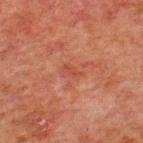Q: Is there a histopathology result?
A: imaged on a skin check; not biopsied
Q: Automated lesion metrics?
A: an outline eccentricity of about 0.85 (0 = round, 1 = elongated); internal color variation of about 1 on a 0–10 scale and a peripheral color-asymmetry measure near 0.5
Q: Who is the patient?
A: male, aged around 60
Q: What kind of image is this?
A: ~15 mm crop, total-body skin-cancer survey
Q: Lesion location?
A: the upper back
Q: How large is the lesion?
A: ≈2.5 mm